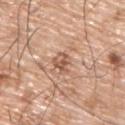The lesion was tiled from a total-body skin photograph and was not biopsied.
The lesion is on the back.
A 15 mm close-up extracted from a 3D total-body photography capture.
A male patient, aged approximately 75.